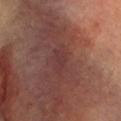Captured during whole-body skin photography for melanoma surveillance; the lesion was not biopsied.
A 15 mm close-up tile from a total-body photography series done for melanoma screening.
Automated image analysis of the tile measured a shape eccentricity near 0.85 and two-axis asymmetry of about 0.4. The analysis additionally found lesion-presence confidence of about 90/100.
On the head or neck.
Imaged with cross-polarized lighting.
A male patient aged 68–72.
Measured at roughly 2.5 mm in maximum diameter.